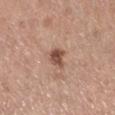  biopsy_status: not biopsied; imaged during a skin examination
  automated_metrics:
    area_mm2_approx: 4.5
    shape_asymmetry: 0.25
    color_variation_0_10: 3.0
    peripheral_color_asymmetry: 1.0
  patient:
    sex: female
    age_approx: 65
  lesion_size:
    long_diameter_mm_approx: 2.5
  image:
    source: total-body photography crop
    field_of_view_mm: 15
  lighting: white-light
  site: leg